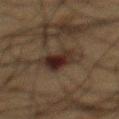biopsy status = imaged on a skin check; not biopsied | acquisition = total-body-photography crop, ~15 mm field of view | site = the back | patient = male, aged approximately 60.